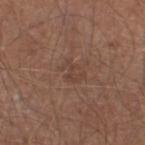Part of a total-body skin-imaging series; this lesion was reviewed on a skin check and was not flagged for biopsy.
The patient is a male approximately 65 years of age.
The recorded lesion diameter is about 2.5 mm.
The lesion is on the left lower leg.
A 15 mm close-up extracted from a 3D total-body photography capture.
Captured under white-light illumination.